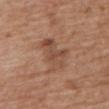On the mid back. Approximately 4.5 mm at its widest. A female patient, aged 73–77. Imaged with white-light lighting. A region of skin cropped from a whole-body photographic capture, roughly 15 mm wide. The total-body-photography lesion software estimated a footprint of about 12 mm², an eccentricity of roughly 0.6, and a shape-asymmetry score of about 0.35 (0 = symmetric). It also reported an average lesion color of about L≈49 a*≈20 b*≈30 (CIELAB) and roughly 8 lightness units darker than nearby skin. The software also gave radial color variation of about 1.5.